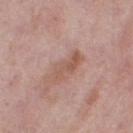notes = catalogued during a skin exam; not biopsied
image source = ~15 mm tile from a whole-body skin photo
size = ≈4.5 mm
illumination = white-light illumination
anatomic site = the right thigh
subject = female, approximately 50 years of age